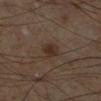A male patient, aged 58–62. Cropped from a total-body skin-imaging series; the visible field is about 15 mm. The tile uses cross-polarized illumination. The lesion is on the leg. The recorded lesion diameter is about 2.5 mm.